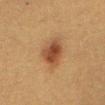<lesion>
  <biopsy_status>not biopsied; imaged during a skin examination</biopsy_status>
  <patient>
    <sex>female</sex>
    <age_approx>40</age_approx>
  </patient>
  <image>
    <source>total-body photography crop</source>
    <field_of_view_mm>15</field_of_view_mm>
  </image>
  <lesion_size>
    <long_diameter_mm_approx>3.5</long_diameter_mm_approx>
  </lesion_size>
  <lighting>cross-polarized</lighting>
  <site>abdomen</site>
</lesion>